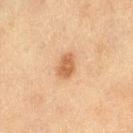body site: the right thigh
image source: ~15 mm crop, total-body skin-cancer survey
patient: female, approximately 55 years of age